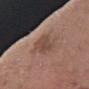Clinical impression:
This lesion was catalogued during total-body skin photography and was not selected for biopsy.
Clinical summary:
A male patient aged 58 to 62. Located on the right forearm. A close-up tile cropped from a whole-body skin photograph, about 15 mm across.